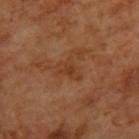| key | value |
|---|---|
| notes | no biopsy performed (imaged during a skin exam) |
| body site | the upper back |
| lighting | cross-polarized illumination |
| subject | male, roughly 65 years of age |
| lesion diameter | ~3 mm (longest diameter) |
| acquisition | ~15 mm tile from a whole-body skin photo |